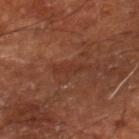No biopsy was performed on this lesion — it was imaged during a full skin examination and was not determined to be concerning. The lesion is on the right lower leg. A subject aged 63 to 67. Automated image analysis of the tile measured a mean CIELAB color near L≈33 a*≈24 b*≈29, a lesion–skin lightness drop of about 5, and a normalized border contrast of about 5. The software also gave an automated nevus-likeness rating near 0 out of 100 and lesion-presence confidence of about 95/100. A 15 mm crop from a total-body photograph taken for skin-cancer surveillance.